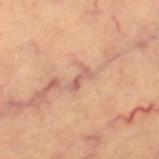The lesion was photographed on a routine skin check and not biopsied; there is no pathology result.
On the right thigh.
A female subject in their mid-60s.
This image is a 15 mm lesion crop taken from a total-body photograph.
About 2.5 mm across.